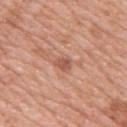<lesion>
  <biopsy_status>not biopsied; imaged during a skin examination</biopsy_status>
  <automated_metrics>
    <area_mm2_approx>3.0</area_mm2_approx>
    <shape_asymmetry>0.3</shape_asymmetry>
    <cielab_L>55</cielab_L>
    <cielab_a>24</cielab_a>
    <cielab_b>30</cielab_b>
    <vs_skin_darker_L>10.0</vs_skin_darker_L>
    <vs_skin_contrast_norm>6.5</vs_skin_contrast_norm>
    <border_irregularity_0_10>2.5</border_irregularity_0_10>
    <color_variation_0_10>2.0</color_variation_0_10>
    <peripheral_color_asymmetry>0.5</peripheral_color_asymmetry>
    <nevus_likeness_0_100>0</nevus_likeness_0_100>
  </automated_metrics>
  <lighting>white-light</lighting>
  <patient>
    <sex>female</sex>
    <age_approx>45</age_approx>
  </patient>
  <site>right upper arm</site>
  <image>
    <source>total-body photography crop</source>
    <field_of_view_mm>15</field_of_view_mm>
  </image>
</lesion>